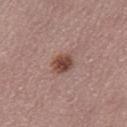acquisition — ~15 mm tile from a whole-body skin photo
subject — female, roughly 55 years of age
automated metrics — a mean CIELAB color near L≈46 a*≈21 b*≈24, about 13 CIELAB-L* units darker than the surrounding skin, and a normalized border contrast of about 9.5; border irregularity of about 1.5 on a 0–10 scale, internal color variation of about 3.5 on a 0–10 scale, and radial color variation of about 1
tile lighting — white-light illumination
site — the leg
lesion size — ~3 mm (longest diameter)